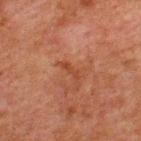{"biopsy_status": "not biopsied; imaged during a skin examination", "patient": {"sex": "male", "age_approx": 60}, "site": "upper back", "automated_metrics": {"area_mm2_approx": 2.0, "eccentricity": 0.95, "shape_asymmetry": 0.55, "nevus_likeness_0_100": 0}, "image": {"source": "total-body photography crop", "field_of_view_mm": 15}}